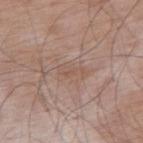No biopsy was performed on this lesion — it was imaged during a full skin examination and was not determined to be concerning.
The lesion is on the right upper arm.
A 15 mm crop from a total-body photograph taken for skin-cancer surveillance.
The lesion-visualizer software estimated an automated nevus-likeness rating near 0 out of 100 and lesion-presence confidence of about 100/100.
The tile uses white-light illumination.
The subject is a male about 75 years old.